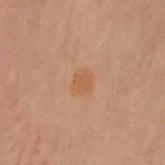{
  "biopsy_status": "not biopsied; imaged during a skin examination",
  "patient": {
    "sex": "female",
    "age_approx": 60
  },
  "site": "left arm",
  "image": {
    "source": "total-body photography crop",
    "field_of_view_mm": 15
  }
}